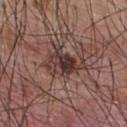Imaged during a routine full-body skin examination; the lesion was not biopsied and no histopathology is available. A 15 mm crop from a total-body photograph taken for skin-cancer surveillance. Captured under white-light illumination. The lesion is located on the upper back. The patient is a male aged 53–57.